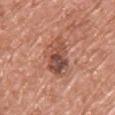biopsy status: no biopsy performed (imaged during a skin exam) | size: ~5 mm (longest diameter) | tile lighting: white-light illumination | automated metrics: a footprint of about 13 mm², an eccentricity of roughly 0.8, and a shape-asymmetry score of about 0.2 (0 = symmetric) | image source: 15 mm crop, total-body photography | subject: male, approximately 70 years of age | anatomic site: the front of the torso.